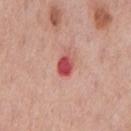notes: imaged on a skin check; not biopsied
lesion size: ≈3 mm
anatomic site: the chest
tile lighting: white-light illumination
subject: male, aged approximately 75
acquisition: total-body-photography crop, ~15 mm field of view